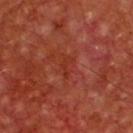Assessment:
No biopsy was performed on this lesion — it was imaged during a full skin examination and was not determined to be concerning.
Background:
The lesion-visualizer software estimated a mean CIELAB color near L≈33 a*≈32 b*≈32, roughly 5 lightness units darker than nearby skin, and a lesion-to-skin contrast of about 5.5 (normalized; higher = more distinct). It also reported a border-irregularity rating of about 5.5/10, internal color variation of about 0 on a 0–10 scale, and peripheral color asymmetry of about 0. A 15 mm close-up extracted from a 3D total-body photography capture. On the front of the torso. The patient is a male about 65 years old.